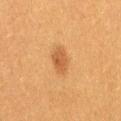follow-up: catalogued during a skin exam; not biopsied
image source: 15 mm crop, total-body photography
automated metrics: peripheral color asymmetry of about 1; an automated nevus-likeness rating near 95 out of 100 and a lesion-detection confidence of about 100/100
body site: the right thigh
patient: female, aged 38 to 42
size: ~3 mm (longest diameter)